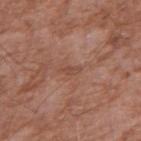Impression:
No biopsy was performed on this lesion — it was imaged during a full skin examination and was not determined to be concerning.
Acquisition and patient details:
A male patient, aged 58–62. Approximately 2.5 mm at its widest. A 15 mm close-up extracted from a 3D total-body photography capture. On the arm. The tile uses white-light illumination. The lesion-visualizer software estimated a footprint of about 2.5 mm², a shape eccentricity near 0.9, and a shape-asymmetry score of about 0.35 (0 = symmetric). The software also gave a lesion–skin lightness drop of about 7. And it measured a classifier nevus-likeness of about 0/100 and a detector confidence of about 100 out of 100 that the crop contains a lesion.